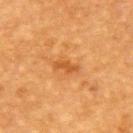workup: catalogued during a skin exam; not biopsied | subject: female, about 55 years old | image source: 15 mm crop, total-body photography | location: the upper back.